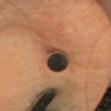<lesion>
  <biopsy_status>not biopsied; imaged during a skin examination</biopsy_status>
  <lighting>cross-polarized</lighting>
  <patient>
    <sex>female</sex>
    <age_approx>50</age_approx>
  </patient>
  <site>head or neck</site>
  <image>
    <source>total-body photography crop</source>
    <field_of_view_mm>15</field_of_view_mm>
  </image>
</lesion>